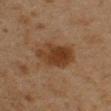Impression: No biopsy was performed on this lesion — it was imaged during a full skin examination and was not determined to be concerning. Clinical summary: Captured under cross-polarized illumination. A 15 mm close-up tile from a total-body photography series done for melanoma screening. Located on the arm. Approximately 5 mm at its widest. A male subject in their mid-40s.